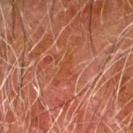The lesion is located on the right forearm. A close-up tile cropped from a whole-body skin photograph, about 15 mm across. Imaged with cross-polarized lighting. The patient is a male aged approximately 80. Longest diameter approximately 2.5 mm.